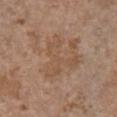Findings:
- biopsy status · no biopsy performed (imaged during a skin exam)
- patient · female, in their mid- to late 60s
- anatomic site · the chest
- imaging modality · 15 mm crop, total-body photography
- lesion size · ≈5.5 mm
- TBP lesion metrics · a classifier nevus-likeness of about 0/100